A 15 mm crop from a total-body photograph taken for skin-cancer surveillance, the patient is a male approximately 45 years of age, from the upper back:
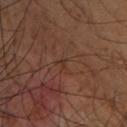Captured under cross-polarized illumination. About 1 mm across. The lesion was biopsied, and histopathology showed a seborrheic keratosis.